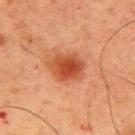Captured during whole-body skin photography for melanoma surveillance; the lesion was not biopsied.